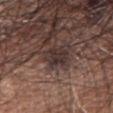  biopsy_status: not biopsied; imaged during a skin examination
  site: head or neck
  patient:
    sex: female
    age_approx: 75
  image:
    source: total-body photography crop
    field_of_view_mm: 15